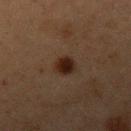| field | value |
|---|---|
| notes | total-body-photography surveillance lesion; no biopsy |
| image source | total-body-photography crop, ~15 mm field of view |
| automated lesion analysis | a lesion area of about 4 mm², an eccentricity of roughly 0.65, and two-axis asymmetry of about 0.15; a mean CIELAB color near L≈19 a*≈16 b*≈21, a lesion–skin lightness drop of about 10, and a normalized border contrast of about 12.5; border irregularity of about 1.5 on a 0–10 scale, internal color variation of about 3 on a 0–10 scale, and a peripheral color-asymmetry measure near 1 |
| subject | female, aged 38 to 42 |
| lesion size | ~2.5 mm (longest diameter) |
| location | the left upper arm |
| tile lighting | cross-polarized |